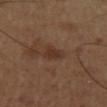notes: imaged on a skin check; not biopsied | site: the left upper arm | subject: male, aged around 50 | image: ~15 mm crop, total-body skin-cancer survey | tile lighting: cross-polarized | TBP lesion metrics: a footprint of about 3.5 mm², an outline eccentricity of about 0.65 (0 = round, 1 = elongated), and a symmetry-axis asymmetry near 0.4 | lesion size: ~2.5 mm (longest diameter).